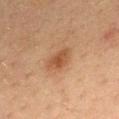About 3.5 mm across.
On the front of the torso.
A female subject aged 53–57.
Imaged with cross-polarized lighting.
A 15 mm close-up extracted from a 3D total-body photography capture.